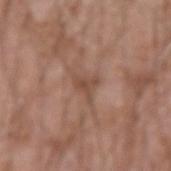Q: Is there a histopathology result?
A: total-body-photography surveillance lesion; no biopsy
Q: What did automated image analysis measure?
A: a normalized border contrast of about 6
Q: Illumination type?
A: white-light illumination
Q: What is the anatomic site?
A: the arm
Q: What are the patient's age and sex?
A: male, about 60 years old
Q: What is the lesion's diameter?
A: ~3 mm (longest diameter)
Q: How was this image acquired?
A: total-body-photography crop, ~15 mm field of view